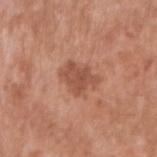Measured at roughly 4 mm in maximum diameter. A 15 mm close-up extracted from a 3D total-body photography capture. The lesion is on the right upper arm. A male patient, aged 53 to 57. Captured under white-light illumination. The total-body-photography lesion software estimated a shape eccentricity near 0.7 and a shape-asymmetry score of about 0.3 (0 = symmetric). The software also gave an average lesion color of about L≈52 a*≈24 b*≈31 (CIELAB), a lesion–skin lightness drop of about 10, and a normalized border contrast of about 6.5.